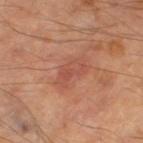Impression: Recorded during total-body skin imaging; not selected for excision or biopsy. Context: The subject is a male aged approximately 65. Cropped from a total-body skin-imaging series; the visible field is about 15 mm. About 4 mm across. Located on the right thigh. This is a cross-polarized tile.